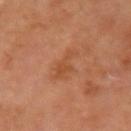Captured during whole-body skin photography for melanoma surveillance; the lesion was not biopsied. Approximately 3.5 mm at its widest. A 15 mm close-up tile from a total-body photography series done for melanoma screening. A female subject, in their mid-50s. This is a cross-polarized tile. The lesion is located on the right upper arm.